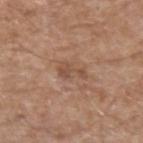<tbp_lesion>
<biopsy_status>not biopsied; imaged during a skin examination</biopsy_status>
<automated_metrics>
  <vs_skin_darker_L>8.0</vs_skin_darker_L>
  <vs_skin_contrast_norm>6.0</vs_skin_contrast_norm>
  <border_irregularity_0_10>6.5</border_irregularity_0_10>
  <color_variation_0_10>1.0</color_variation_0_10>
  <peripheral_color_asymmetry>0.5</peripheral_color_asymmetry>
</automated_metrics>
<site>left upper arm</site>
<image>
  <source>total-body photography crop</source>
  <field_of_view_mm>15</field_of_view_mm>
</image>
<patient>
  <sex>male</sex>
  <age_approx>60</age_approx>
</patient>
</tbp_lesion>